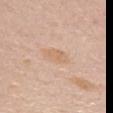No biopsy was performed on this lesion — it was imaged during a full skin examination and was not determined to be concerning.
This image is a 15 mm lesion crop taken from a total-body photograph.
The patient is a female roughly 60 years of age.
On the chest.